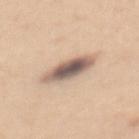Part of a total-body skin-imaging series; this lesion was reviewed on a skin check and was not flagged for biopsy.
Located on the back.
The recorded lesion diameter is about 5.5 mm.
A 15 mm crop from a total-body photograph taken for skin-cancer surveillance.
Captured under white-light illumination.
A female subject roughly 30 years of age.